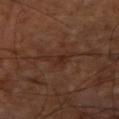Imaged during a routine full-body skin examination; the lesion was not biopsied and no histopathology is available. About 3 mm across. Automated tile analysis of the lesion measured an area of roughly 4 mm², a shape eccentricity near 0.8, and a symmetry-axis asymmetry near 0.6. And it measured a color-variation rating of about 2/10 and peripheral color asymmetry of about 0.5. This is a cross-polarized tile. The lesion is on the arm. The subject is aged 63 to 67. A 15 mm close-up tile from a total-body photography series done for melanoma screening.